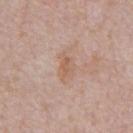biopsy status: catalogued during a skin exam; not biopsied
TBP lesion metrics: an eccentricity of roughly 0.75 and a shape-asymmetry score of about 0.35 (0 = symmetric); a normalized border contrast of about 5.5; a border-irregularity index near 3/10, internal color variation of about 2.5 on a 0–10 scale, and a peripheral color-asymmetry measure near 1
lighting: white-light
patient: male, aged approximately 70
image source: ~15 mm crop, total-body skin-cancer survey
body site: the abdomen